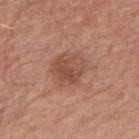  image:
    source: total-body photography crop
    field_of_view_mm: 15
  patient:
    sex: male
    age_approx: 55
  site: arm
  lighting: white-light
  lesion_size:
    long_diameter_mm_approx: 5.0